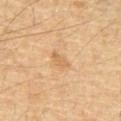The lesion is on the front of the torso.
A male patient aged 63 to 67.
A 15 mm crop from a total-body photograph taken for skin-cancer surveillance.